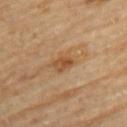Part of a total-body skin-imaging series; this lesion was reviewed on a skin check and was not flagged for biopsy. Automated image analysis of the tile measured an average lesion color of about L≈48 a*≈20 b*≈36 (CIELAB), about 9 CIELAB-L* units darker than the surrounding skin, and a normalized border contrast of about 7.5. The software also gave border irregularity of about 2.5 on a 0–10 scale, internal color variation of about 4 on a 0–10 scale, and radial color variation of about 1.5. It also reported a classifier nevus-likeness of about 0/100 and a lesion-detection confidence of about 100/100. Captured under cross-polarized illumination. A lesion tile, about 15 mm wide, cut from a 3D total-body photograph. A male patient aged around 85 or older. From the upper back. About 2.5 mm across.